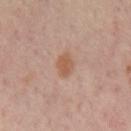Captured during whole-body skin photography for melanoma surveillance; the lesion was not biopsied.
A 15 mm close-up tile from a total-body photography series done for melanoma screening.
A subject about 55 years old.
Imaged with cross-polarized lighting.
Located on the mid back.
Automated tile analysis of the lesion measured a border-irregularity index near 2.5/10 and radial color variation of about 0.5.